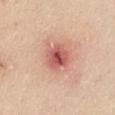| key | value |
|---|---|
| notes | imaged on a skin check; not biopsied |
| location | the right upper arm |
| image | total-body-photography crop, ~15 mm field of view |
| subject | male, in their mid-60s |